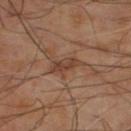  biopsy_status: not biopsied; imaged during a skin examination
  image:
    source: total-body photography crop
    field_of_view_mm: 15
  site: left lower leg
  automated_metrics:
    area_mm2_approx: 5.0
    eccentricity: 0.85
    shape_asymmetry: 0.3
    color_variation_0_10: 3.0
    peripheral_color_asymmetry: 1.0
    nevus_likeness_0_100: 0
    lesion_detection_confidence_0_100: 95
  patient:
    sex: male
    age_approx: 70